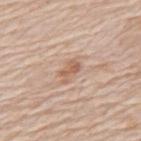biopsy status: imaged on a skin check; not biopsied | image source: total-body-photography crop, ~15 mm field of view | location: the mid back | subject: male, roughly 80 years of age.